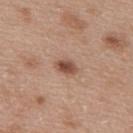Assessment: No biopsy was performed on this lesion — it was imaged during a full skin examination and was not determined to be concerning. Context: A region of skin cropped from a whole-body photographic capture, roughly 15 mm wide. A female subject, approximately 40 years of age. Approximately 3 mm at its widest. On the upper back. Captured under white-light illumination.